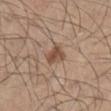biopsy status — no biopsy performed (imaged during a skin exam) | subject — male, aged 43 to 47 | acquisition — ~15 mm tile from a whole-body skin photo | lighting — white-light | body site — the right lower leg.